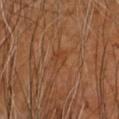Captured during whole-body skin photography for melanoma surveillance; the lesion was not biopsied. On the arm. A male patient roughly 60 years of age. This image is a 15 mm lesion crop taken from a total-body photograph. Captured under cross-polarized illumination. The total-body-photography lesion software estimated an area of roughly 4 mm², an eccentricity of roughly 0.75, and two-axis asymmetry of about 0.3. It also reported border irregularity of about 2.5 on a 0–10 scale and radial color variation of about 1.5.